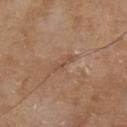Q: Was this lesion biopsied?
A: total-body-photography surveillance lesion; no biopsy
Q: What is the imaging modality?
A: ~15 mm tile from a whole-body skin photo
Q: Who is the patient?
A: male, in their mid-60s
Q: How large is the lesion?
A: about 2.5 mm
Q: Where on the body is the lesion?
A: the left lower leg
Q: What lighting was used for the tile?
A: cross-polarized illumination